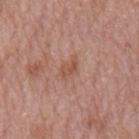Acquisition and patient details:
On the mid back. A male subject, aged 63–67. Measured at roughly 2.5 mm in maximum diameter. Captured under white-light illumination. A 15 mm close-up tile from a total-body photography series done for melanoma screening.